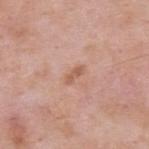Notes:
* workup — total-body-photography surveillance lesion; no biopsy